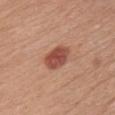<tbp_lesion>
  <biopsy_status>not biopsied; imaged during a skin examination</biopsy_status>
  <patient>
    <sex>female</sex>
    <age_approx>55</age_approx>
  </patient>
  <lighting>white-light</lighting>
  <automated_metrics>
    <eccentricity>0.7</eccentricity>
    <shape_asymmetry>0.2</shape_asymmetry>
    <vs_skin_darker_L>13.0</vs_skin_darker_L>
    <vs_skin_contrast_norm>9.0</vs_skin_contrast_norm>
  </automated_metrics>
  <image>
    <source>total-body photography crop</source>
    <field_of_view_mm>15</field_of_view_mm>
  </image>
  <lesion_size>
    <long_diameter_mm_approx>4.0</long_diameter_mm_approx>
  </lesion_size>
  <site>chest</site>
</tbp_lesion>